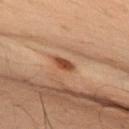Imaged during a routine full-body skin examination; the lesion was not biopsied and no histopathology is available.
The lesion is located on the back.
Cropped from a total-body skin-imaging series; the visible field is about 15 mm.
Longest diameter approximately 3 mm.
A male patient, in their 50s.
The tile uses cross-polarized illumination.